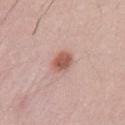Part of a total-body skin-imaging series; this lesion was reviewed on a skin check and was not flagged for biopsy.